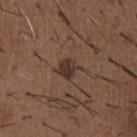<tbp_lesion>
  <biopsy_status>not biopsied; imaged during a skin examination</biopsy_status>
  <image>
    <source>total-body photography crop</source>
    <field_of_view_mm>15</field_of_view_mm>
  </image>
  <automated_metrics>
    <cielab_L>33</cielab_L>
    <cielab_a>16</cielab_a>
    <cielab_b>22</cielab_b>
    <vs_skin_darker_L>9.0</vs_skin_darker_L>
    <vs_skin_contrast_norm>9.0</vs_skin_contrast_norm>
    <color_variation_0_10>2.5</color_variation_0_10>
    <peripheral_color_asymmetry>1.0</peripheral_color_asymmetry>
    <nevus_likeness_0_100>100</nevus_likeness_0_100>
    <lesion_detection_confidence_0_100>100</lesion_detection_confidence_0_100>
  </automated_metrics>
  <site>chest</site>
  <patient>
    <sex>male</sex>
    <age_approx>50</age_approx>
  </patient>
  <lesion_size>
    <long_diameter_mm_approx>2.5</long_diameter_mm_approx>
  </lesion_size>
</tbp_lesion>